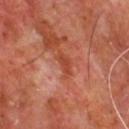Notes:
* biopsy status · catalogued during a skin exam; not biopsied
* acquisition · total-body-photography crop, ~15 mm field of view
* patient · male, aged 63–67
* site · the front of the torso